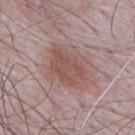The lesion was photographed on a routine skin check and not biopsied; there is no pathology result.
The lesion is located on the mid back.
A region of skin cropped from a whole-body photographic capture, roughly 15 mm wide.
The lesion's longest dimension is about 5 mm.
This is a white-light tile.
The subject is a male aged around 65.